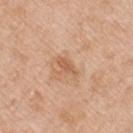<case>
<biopsy_status>not biopsied; imaged during a skin examination</biopsy_status>
<lesion_size>
  <long_diameter_mm_approx>3.0</long_diameter_mm_approx>
</lesion_size>
<site>left upper arm</site>
<automated_metrics>
  <area_mm2_approx>3.5</area_mm2_approx>
  <eccentricity>0.9</eccentricity>
  <shape_asymmetry>0.35</shape_asymmetry>
  <cielab_L>59</cielab_L>
  <cielab_a>22</cielab_a>
  <cielab_b>35</cielab_b>
  <vs_skin_darker_L>8.0</vs_skin_darker_L>
  <vs_skin_contrast_norm>6.0</vs_skin_contrast_norm>
  <lesion_detection_confidence_0_100>100</lesion_detection_confidence_0_100>
</automated_metrics>
<patient>
  <sex>male</sex>
  <age_approx>50</age_approx>
</patient>
<lighting>white-light</lighting>
<image>
  <source>total-body photography crop</source>
  <field_of_view_mm>15</field_of_view_mm>
</image>
</case>